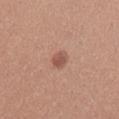notes = total-body-photography surveillance lesion; no biopsy
automated metrics = a border-irregularity index near 2/10, internal color variation of about 2.5 on a 0–10 scale, and a peripheral color-asymmetry measure near 0.5; a classifier nevus-likeness of about 85/100
image source = ~15 mm tile from a whole-body skin photo
tile lighting = white-light illumination
anatomic site = the chest
patient = female, aged approximately 25